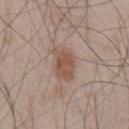follow-up = no biopsy performed (imaged during a skin exam); image source = total-body-photography crop, ~15 mm field of view; site = the chest; patient = male, in their 50s.